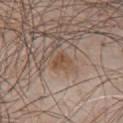Assessment:
This lesion was catalogued during total-body skin photography and was not selected for biopsy.
Background:
Cropped from a whole-body photographic skin survey; the tile spans about 15 mm. A male patient in their mid- to late 50s. On the chest. This is a white-light tile.